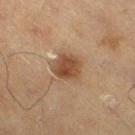location — the right thigh | tile lighting — cross-polarized illumination | TBP lesion metrics — a footprint of about 8 mm², a shape eccentricity near 0.55, and two-axis asymmetry of about 0.25; a mean CIELAB color near L≈38 a*≈16 b*≈27 and a normalized border contrast of about 9; a border-irregularity rating of about 2/10 and peripheral color asymmetry of about 1; an automated nevus-likeness rating near 75 out of 100 and a detector confidence of about 100 out of 100 that the crop contains a lesion | patient — male, aged 68–72 | imaging modality — total-body-photography crop, ~15 mm field of view.